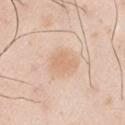This lesion was catalogued during total-body skin photography and was not selected for biopsy. The lesion is located on the arm. The total-body-photography lesion software estimated an automated nevus-likeness rating near 30 out of 100 and a detector confidence of about 100 out of 100 that the crop contains a lesion. The tile uses white-light illumination. About 2.5 mm across. A male patient, roughly 40 years of age. A 15 mm close-up extracted from a 3D total-body photography capture.